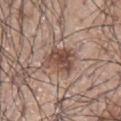biopsy_status: not biopsied; imaged during a skin examination
patient:
  sex: male
  age_approx: 70
image:
  source: total-body photography crop
  field_of_view_mm: 15
site: left arm
lighting: white-light
lesion_size:
  long_diameter_mm_approx: 4.5